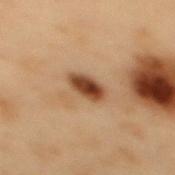biopsy status = no biopsy performed (imaged during a skin exam) | diameter = ~3 mm (longest diameter) | image-analysis metrics = an area of roughly 5.5 mm², an eccentricity of roughly 0.75, and two-axis asymmetry of about 0.15; a lesion–skin lightness drop of about 14; a border-irregularity index near 1.5/10, a within-lesion color-variation index near 4.5/10, and peripheral color asymmetry of about 1.5; a nevus-likeness score of about 100/100 | image = 15 mm crop, total-body photography | lighting = cross-polarized | site = the back | subject = male, aged 53–57.